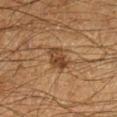{
  "biopsy_status": "not biopsied; imaged during a skin examination",
  "lesion_size": {
    "long_diameter_mm_approx": 3.0
  },
  "automated_metrics": {
    "area_mm2_approx": 6.0,
    "eccentricity": 0.7,
    "shape_asymmetry": 0.25,
    "cielab_L": 35,
    "cielab_a": 16,
    "cielab_b": 28,
    "vs_skin_contrast_norm": 8.5,
    "nevus_likeness_0_100": 60,
    "lesion_detection_confidence_0_100": 100
  },
  "patient": {
    "sex": "male",
    "age_approx": 65
  },
  "image": {
    "source": "total-body photography crop",
    "field_of_view_mm": 15
  },
  "site": "left lower leg"
}